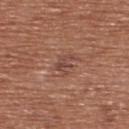No biopsy was performed on this lesion — it was imaged during a full skin examination and was not determined to be concerning.
On the upper back.
The subject is a male roughly 65 years of age.
A lesion tile, about 15 mm wide, cut from a 3D total-body photograph.